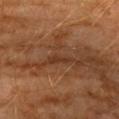follow-up=imaged on a skin check; not biopsied
tile lighting=cross-polarized
acquisition=15 mm crop, total-body photography
patient=male, about 60 years old
location=the left upper arm
lesion diameter=~3 mm (longest diameter)
automated metrics=a footprint of about 2.5 mm², a shape eccentricity near 0.95, and a symmetry-axis asymmetry near 0.4; roughly 6 lightness units darker than nearby skin and a normalized border contrast of about 6; a classifier nevus-likeness of about 0/100